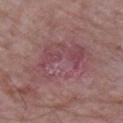| field | value |
|---|---|
| notes | no biopsy performed (imaged during a skin exam) |
| patient | male, about 65 years old |
| tile lighting | white-light |
| image source | ~15 mm crop, total-body skin-cancer survey |
| site | the right upper arm |
| automated lesion analysis | a mean CIELAB color near L≈47 a*≈23 b*≈16, roughly 6 lightness units darker than nearby skin, and a normalized border contrast of about 5; an automated nevus-likeness rating near 0 out of 100 and a lesion-detection confidence of about 80/100 |